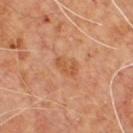Q: Was a biopsy performed?
A: catalogued during a skin exam; not biopsied
Q: How large is the lesion?
A: ≈3 mm
Q: Patient demographics?
A: male, aged approximately 60
Q: Where on the body is the lesion?
A: the upper back
Q: What is the imaging modality?
A: ~15 mm crop, total-body skin-cancer survey
Q: What lighting was used for the tile?
A: cross-polarized illumination